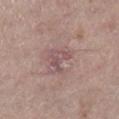follow-up: no biopsy performed (imaged during a skin exam)
illumination: white-light illumination
body site: the right lower leg
imaging modality: ~15 mm crop, total-body skin-cancer survey
TBP lesion metrics: a lesion area of about 4.5 mm², an outline eccentricity of about 0.35 (0 = round, 1 = elongated), and a shape-asymmetry score of about 0.6 (0 = symmetric); a lesion color around L≈52 a*≈19 b*≈18 in CIELAB and a normalized lesion–skin contrast near 6; a nevus-likeness score of about 0/100 and a detector confidence of about 90 out of 100 that the crop contains a lesion
patient: female, aged 63 to 67
diameter: about 2.5 mm